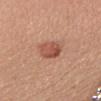Q: Was a biopsy performed?
A: no biopsy performed (imaged during a skin exam)
Q: What is the lesion's diameter?
A: ≈3 mm
Q: What did automated image analysis measure?
A: a lesion area of about 7 mm², an eccentricity of roughly 0.6, and a shape-asymmetry score of about 0.15 (0 = symmetric); a mean CIELAB color near L≈53 a*≈26 b*≈31 and a lesion–skin lightness drop of about 11
Q: What kind of image is this?
A: total-body-photography crop, ~15 mm field of view
Q: What lighting was used for the tile?
A: white-light
Q: Who is the patient?
A: female, aged approximately 45
Q: Lesion location?
A: the chest